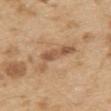location: the upper back | patient: male, roughly 70 years of age | lighting: white-light | imaging modality: ~15 mm crop, total-body skin-cancer survey | size: ~5 mm (longest diameter).